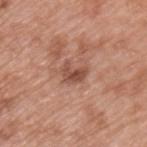Findings:
- image: 15 mm crop, total-body photography
- body site: the back
- patient: male, about 50 years old